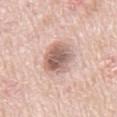image-analysis metrics — a shape-asymmetry score of about 0.15 (0 = symmetric); an average lesion color of about L≈61 a*≈19 b*≈24 (CIELAB) and roughly 15 lightness units darker than nearby skin; a border-irregularity index near 1.5/10 and a within-lesion color-variation index near 5.5/10; an automated nevus-likeness rating near 30 out of 100 | body site — the front of the torso | acquisition — ~15 mm crop, total-body skin-cancer survey | patient — male, approximately 65 years of age | lesion diameter — about 4.5 mm.